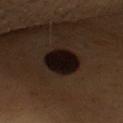follow-up — total-body-photography surveillance lesion; no biopsy
subject — male, aged 63–67
image — ~15 mm tile from a whole-body skin photo
size — ≈4.5 mm
anatomic site — the chest
illumination — cross-polarized illumination